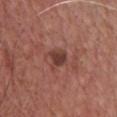Imaged during a routine full-body skin examination; the lesion was not biopsied and no histopathology is available. About 2.5 mm across. A 15 mm close-up tile from a total-body photography series done for melanoma screening. The lesion-visualizer software estimated internal color variation of about 2.5 on a 0–10 scale and peripheral color asymmetry of about 0.5. A male subject aged approximately 65. This is a white-light tile. The lesion is located on the front of the torso.